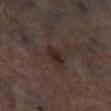Clinical summary:
A roughly 15 mm field-of-view crop from a total-body skin photograph. The lesion is located on the leg. The patient is a male aged 63–67.